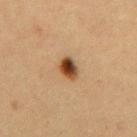Notes:
- notes · imaged on a skin check; not biopsied